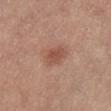No biopsy was performed on this lesion — it was imaged during a full skin examination and was not determined to be concerning.
The lesion is on the abdomen.
A female patient, aged around 45.
This image is a 15 mm lesion crop taken from a total-body photograph.
Measured at roughly 3 mm in maximum diameter.
The tile uses white-light illumination.
Automated image analysis of the tile measured an area of roughly 6 mm², an eccentricity of roughly 0.6, and a symmetry-axis asymmetry near 0.2. The software also gave a mean CIELAB color near L≈51 a*≈24 b*≈28 and roughly 9 lightness units darker than nearby skin. The software also gave a nevus-likeness score of about 75/100 and lesion-presence confidence of about 100/100.